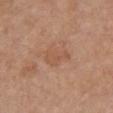Assessment:
No biopsy was performed on this lesion — it was imaged during a full skin examination and was not determined to be concerning.
Context:
A female patient aged 53 to 57. A 15 mm close-up extracted from a 3D total-body photography capture. The tile uses white-light illumination. Measured at roughly 3.5 mm in maximum diameter. From the chest.